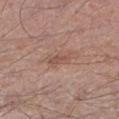– image · ~15 mm crop, total-body skin-cancer survey
– lesion size · ≈3.5 mm
– automated metrics · two-axis asymmetry of about 0.35; internal color variation of about 2.5 on a 0–10 scale and radial color variation of about 1; a nevus-likeness score of about 0/100
– patient · male, in their mid- to late 50s
– anatomic site · the left lower leg
– lighting · white-light